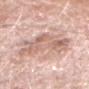{
  "biopsy_status": "not biopsied; imaged during a skin examination",
  "site": "head or neck",
  "patient": {
    "sex": "female",
    "age_approx": 75
  },
  "image": {
    "source": "total-body photography crop",
    "field_of_view_mm": 15
  },
  "lighting": "white-light"
}